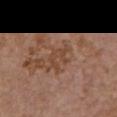Q: Was this lesion biopsied?
A: catalogued during a skin exam; not biopsied
Q: Where on the body is the lesion?
A: the front of the torso
Q: What is the lesion's diameter?
A: ≈4 mm
Q: Who is the patient?
A: female, roughly 65 years of age
Q: What is the imaging modality?
A: ~15 mm tile from a whole-body skin photo
Q: How was the tile lit?
A: white-light